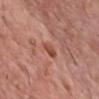No biopsy was performed on this lesion — it was imaged during a full skin examination and was not determined to be concerning.
The lesion is located on the front of the torso.
Cropped from a total-body skin-imaging series; the visible field is about 15 mm.
A male patient, roughly 60 years of age.